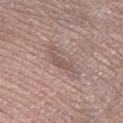The lesion was tiled from a total-body skin photograph and was not biopsied.
On the right lower leg.
Longest diameter approximately 5 mm.
Captured under white-light illumination.
A 15 mm close-up extracted from a 3D total-body photography capture.
The patient is a male in their mid-50s.
Automated tile analysis of the lesion measured an area of roughly 7 mm² and a symmetry-axis asymmetry near 0.45. The analysis additionally found a lesion–skin lightness drop of about 8. And it measured a border-irregularity rating of about 6/10, internal color variation of about 1.5 on a 0–10 scale, and peripheral color asymmetry of about 0.5. The software also gave a classifier nevus-likeness of about 0/100 and a detector confidence of about 60 out of 100 that the crop contains a lesion.